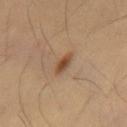follow-up=catalogued during a skin exam; not biopsied
acquisition=total-body-photography crop, ~15 mm field of view
site=the mid back
lighting=cross-polarized
diameter=about 3 mm
patient=male, aged 53 to 57